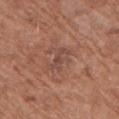Impression: No biopsy was performed on this lesion — it was imaged during a full skin examination and was not determined to be concerning. Acquisition and patient details: Imaged with white-light lighting. The lesion-visualizer software estimated an average lesion color of about L≈46 a*≈21 b*≈25 (CIELAB), a lesion–skin lightness drop of about 6, and a normalized border contrast of about 5.5. And it measured a nevus-likeness score of about 0/100 and a detector confidence of about 100 out of 100 that the crop contains a lesion. This image is a 15 mm lesion crop taken from a total-body photograph. The lesion is located on the upper back. A female subject aged around 75. Measured at roughly 3.5 mm in maximum diameter.